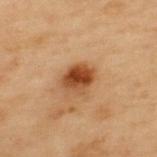biopsy_status: not biopsied; imaged during a skin examination
site: upper back
lesion_size:
  long_diameter_mm_approx: 3.5
patient:
  sex: male
  age_approx: 55
automated_metrics:
  area_mm2_approx: 7.5
  shape_asymmetry: 0.15
  border_irregularity_0_10: 1.5
  color_variation_0_10: 5.5
  peripheral_color_asymmetry: 2.0
  nevus_likeness_0_100: 95
  lesion_detection_confidence_0_100: 100
lighting: cross-polarized
image:
  source: total-body photography crop
  field_of_view_mm: 15